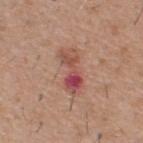Case summary:
– workup — catalogued during a skin exam; not biopsied
– subject — male, approximately 60 years of age
– automated metrics — a footprint of about 8.5 mm², an outline eccentricity of about 0.9 (0 = round, 1 = elongated), and a symmetry-axis asymmetry near 0.25; roughly 11 lightness units darker than nearby skin and a normalized border contrast of about 8; a border-irregularity index near 4.5/10 and radial color variation of about 4
– imaging modality — ~15 mm crop, total-body skin-cancer survey
– location — the back